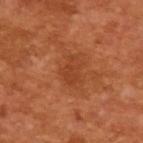biopsy status: no biopsy performed (imaged during a skin exam)
patient: male, in their mid- to late 60s
automated metrics: a color-variation rating of about 1.5/10 and a peripheral color-asymmetry measure near 0.5; a nevus-likeness score of about 0/100 and lesion-presence confidence of about 100/100
lesion size: ~3.5 mm (longest diameter)
illumination: cross-polarized
image source: 15 mm crop, total-body photography